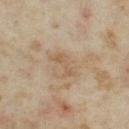  biopsy_status: not biopsied; imaged during a skin examination
  image:
    source: total-body photography crop
    field_of_view_mm: 15
  site: right thigh
  lesion_size:
    long_diameter_mm_approx: 3.0
  patient:
    sex: female
    age_approx: 35
  lighting: cross-polarized